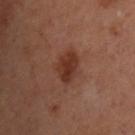workup: total-body-photography surveillance lesion; no biopsy
diameter: ~3.5 mm (longest diameter)
lighting: cross-polarized
image: ~15 mm crop, total-body skin-cancer survey
patient: female, approximately 55 years of age
image-analysis metrics: a lesion area of about 6.5 mm², an eccentricity of roughly 0.65, and a shape-asymmetry score of about 0.25 (0 = symmetric); a border-irregularity rating of about 2.5/10
site: the back